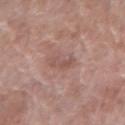Imaged during a routine full-body skin examination; the lesion was not biopsied and no histopathology is available. On the arm. A female subject, in their 60s. A lesion tile, about 15 mm wide, cut from a 3D total-body photograph. About 2.5 mm across. Captured under white-light illumination.